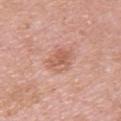The lesion was tiled from a total-body skin photograph and was not biopsied.
An algorithmic analysis of the crop reported a lesion color around L≈60 a*≈24 b*≈30 in CIELAB and a lesion-to-skin contrast of about 6 (normalized; higher = more distinct). It also reported a border-irregularity rating of about 2/10, a color-variation rating of about 4/10, and a peripheral color-asymmetry measure near 1. And it measured a nevus-likeness score of about 10/100.
The subject is a female aged 28–32.
A 15 mm close-up extracted from a 3D total-body photography capture.
On the upper back.
The tile uses white-light illumination.